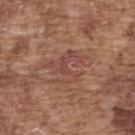Assessment:
Captured during whole-body skin photography for melanoma surveillance; the lesion was not biopsied.
Background:
A male patient, aged 73 to 77. The lesion is on the upper back. Approximately 6 mm at its widest. Imaged with white-light lighting. A roughly 15 mm field-of-view crop from a total-body skin photograph.